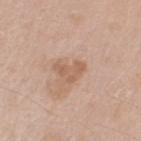Q: Was this lesion biopsied?
A: total-body-photography surveillance lesion; no biopsy
Q: What is the imaging modality?
A: ~15 mm crop, total-body skin-cancer survey
Q: What is the anatomic site?
A: the left upper arm
Q: What is the lesion's diameter?
A: ~3.5 mm (longest diameter)
Q: Patient demographics?
A: male, aged 63–67